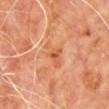Recorded during total-body skin imaging; not selected for excision or biopsy. The lesion is located on the upper back. A region of skin cropped from a whole-body photographic capture, roughly 15 mm wide. A male patient, about 60 years old.